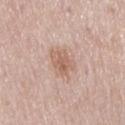Q: Is there a histopathology result?
A: imaged on a skin check; not biopsied
Q: Who is the patient?
A: male, aged 53–57
Q: How was this image acquired?
A: ~15 mm tile from a whole-body skin photo
Q: How large is the lesion?
A: ~3.5 mm (longest diameter)
Q: Automated lesion metrics?
A: an average lesion color of about L≈61 a*≈20 b*≈27 (CIELAB), a lesion–skin lightness drop of about 9, and a lesion-to-skin contrast of about 6.5 (normalized; higher = more distinct)
Q: What is the anatomic site?
A: the mid back